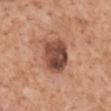Imaged during a routine full-body skin examination; the lesion was not biopsied and no histopathology is available.
Captured under white-light illumination.
A male patient about 60 years old.
Automated image analysis of the tile measured an average lesion color of about L≈47 a*≈22 b*≈28 (CIELAB), about 15 CIELAB-L* units darker than the surrounding skin, and a lesion-to-skin contrast of about 10.5 (normalized; higher = more distinct). The software also gave border irregularity of about 1.5 on a 0–10 scale and a peripheral color-asymmetry measure near 2.5. The software also gave a classifier nevus-likeness of about 70/100.
From the mid back.
Measured at roughly 4 mm in maximum diameter.
A close-up tile cropped from a whole-body skin photograph, about 15 mm across.